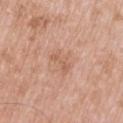The lesion was tiled from a total-body skin photograph and was not biopsied.
Longest diameter approximately 3.5 mm.
The patient is a male approximately 50 years of age.
Imaged with white-light lighting.
The lesion-visualizer software estimated a footprint of about 4.5 mm² and a shape-asymmetry score of about 0.5 (0 = symmetric). The analysis additionally found a mean CIELAB color near L≈60 a*≈21 b*≈32, roughly 7 lightness units darker than nearby skin, and a lesion-to-skin contrast of about 5 (normalized; higher = more distinct). The analysis additionally found a border-irregularity rating of about 7/10, internal color variation of about 0 on a 0–10 scale, and peripheral color asymmetry of about 0. The software also gave a classifier nevus-likeness of about 0/100 and lesion-presence confidence of about 100/100.
Cropped from a total-body skin-imaging series; the visible field is about 15 mm.
From the arm.